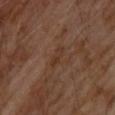{"biopsy_status": "not biopsied; imaged during a skin examination", "lesion_size": {"long_diameter_mm_approx": 2.5}, "image": {"source": "total-body photography crop", "field_of_view_mm": 15}, "patient": {"sex": "male", "age_approx": 70}, "site": "upper back"}